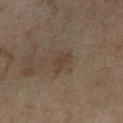Q: Was this lesion biopsied?
A: imaged on a skin check; not biopsied
Q: Who is the patient?
A: female, about 60 years old
Q: What is the imaging modality?
A: 15 mm crop, total-body photography
Q: Where on the body is the lesion?
A: the left lower leg